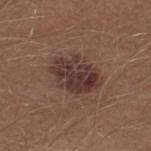Acquisition and patient details:
Longest diameter approximately 5 mm. A male patient roughly 30 years of age. Imaged with white-light lighting. The lesion is located on the leg. An algorithmic analysis of the crop reported internal color variation of about 5 on a 0–10 scale and peripheral color asymmetry of about 2. It also reported an automated nevus-likeness rating near 30 out of 100 and lesion-presence confidence of about 100/100. A roughly 15 mm field-of-view crop from a total-body skin photograph.